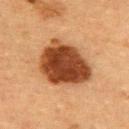Impression: The lesion was photographed on a routine skin check and not biopsied; there is no pathology result. Context: Automated image analysis of the tile measured border irregularity of about 2 on a 0–10 scale, a within-lesion color-variation index near 5/10, and radial color variation of about 1.5. A female subject, approximately 55 years of age. Approximately 6.5 mm at its widest. A 15 mm close-up tile from a total-body photography series done for melanoma screening. The lesion is on the upper back. Captured under cross-polarized illumination.